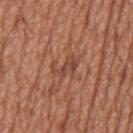Q: Was a biopsy performed?
A: no biopsy performed (imaged during a skin exam)
Q: Who is the patient?
A: male, aged approximately 65
Q: How was this image acquired?
A: ~15 mm tile from a whole-body skin photo
Q: What is the anatomic site?
A: the mid back
Q: How large is the lesion?
A: ~3.5 mm (longest diameter)